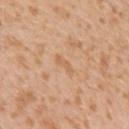Findings:
- biopsy status — no biopsy performed (imaged during a skin exam)
- subject — female, about 30 years old
- lesion size — ≈3 mm
- image source — ~15 mm crop, total-body skin-cancer survey
- site — the left upper arm